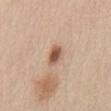biopsy status = total-body-photography surveillance lesion; no biopsy
acquisition = total-body-photography crop, ~15 mm field of view
TBP lesion metrics = a lesion color around L≈55 a*≈20 b*≈30 in CIELAB, a lesion–skin lightness drop of about 15, and a normalized border contrast of about 9.5
anatomic site = the abdomen
patient = male, approximately 60 years of age
size = ~3 mm (longest diameter)